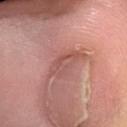Q: Is there a histopathology result?
A: no biopsy performed (imaged during a skin exam)
Q: What is the imaging modality?
A: 15 mm crop, total-body photography
Q: Patient demographics?
A: male, aged around 60
Q: Where on the body is the lesion?
A: the left lower leg
Q: How large is the lesion?
A: about 4.5 mm
Q: Illumination type?
A: white-light illumination
Q: Automated lesion metrics?
A: a border-irregularity rating of about 4.5/10, a within-lesion color-variation index near 3/10, and a peripheral color-asymmetry measure near 1